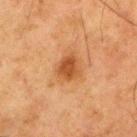follow-up: imaged on a skin check; not biopsied
image source: total-body-photography crop, ~15 mm field of view
anatomic site: the right thigh
lesion size: ≈3 mm
subject: male, aged 78–82
tile lighting: cross-polarized